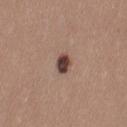Imaged during a routine full-body skin examination; the lesion was not biopsied and no histopathology is available. A region of skin cropped from a whole-body photographic capture, roughly 15 mm wide. A female subject, aged approximately 35. From the left thigh. An algorithmic analysis of the crop reported an outline eccentricity of about 0.6 (0 = round, 1 = elongated) and two-axis asymmetry of about 0.3. Imaged with white-light lighting.